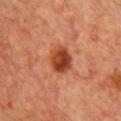{
  "biopsy_status": "not biopsied; imaged during a skin examination",
  "lighting": "cross-polarized",
  "lesion_size": {
    "long_diameter_mm_approx": 3.5
  },
  "image": {
    "source": "total-body photography crop",
    "field_of_view_mm": 15
  },
  "patient": {
    "sex": "female",
    "age_approx": 55
  },
  "site": "front of the torso"
}